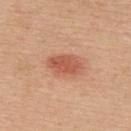– notes — no biopsy performed (imaged during a skin exam)